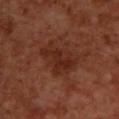Part of a total-body skin-imaging series; this lesion was reviewed on a skin check and was not flagged for biopsy. Cropped from a total-body skin-imaging series; the visible field is about 15 mm. An algorithmic analysis of the crop reported an average lesion color of about L≈26 a*≈24 b*≈26 (CIELAB) and a normalized lesion–skin contrast near 7. On the upper back. A male patient aged around 70. The tile uses cross-polarized illumination. Measured at roughly 5.5 mm in maximum diameter.